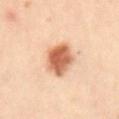* notes — total-body-photography surveillance lesion; no biopsy
* location — the abdomen
* subject — female, aged 28–32
* lesion diameter — ~4.5 mm (longest diameter)
* automated lesion analysis — a shape eccentricity near 0.7 and a shape-asymmetry score of about 0.15 (0 = symmetric); border irregularity of about 1.5 on a 0–10 scale, a color-variation rating of about 4.5/10, and peripheral color asymmetry of about 1.5
* imaging modality — ~15 mm crop, total-body skin-cancer survey
* illumination — cross-polarized illumination